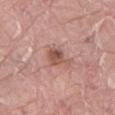{"biopsy_status": "not biopsied; imaged during a skin examination", "patient": {"sex": "male", "age_approx": 40}, "image": {"source": "total-body photography crop", "field_of_view_mm": 15}, "site": "abdomen"}